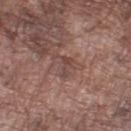Findings:
- workup — total-body-photography surveillance lesion; no biopsy
- illumination — white-light illumination
- anatomic site — the left lower leg
- image — ~15 mm tile from a whole-body skin photo
- patient — male, aged approximately 75
- lesion size — about 2.5 mm